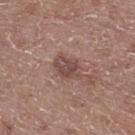Assessment: Imaged during a routine full-body skin examination; the lesion was not biopsied and no histopathology is available. Background: This image is a 15 mm lesion crop taken from a total-body photograph. The lesion is on the back. Measured at roughly 3.5 mm in maximum diameter. Automated tile analysis of the lesion measured a shape-asymmetry score of about 0.25 (0 = symmetric). The analysis additionally found about 9 CIELAB-L* units darker than the surrounding skin and a normalized lesion–skin contrast near 7. The analysis additionally found a border-irregularity rating of about 2.5/10, a within-lesion color-variation index near 3.5/10, and radial color variation of about 1. The patient is a male approximately 60 years of age. This is a white-light tile.